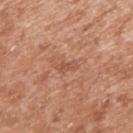Part of a total-body skin-imaging series; this lesion was reviewed on a skin check and was not flagged for biopsy.